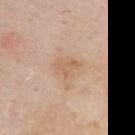Assessment: This lesion was catalogued during total-body skin photography and was not selected for biopsy. Acquisition and patient details: The lesion's longest dimension is about 3.5 mm. On the arm. A male patient aged 63 to 67. Imaged with white-light lighting. Cropped from a whole-body photographic skin survey; the tile spans about 15 mm.